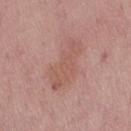- biopsy status — total-body-photography surveillance lesion; no biopsy
- location — the right thigh
- acquisition — total-body-photography crop, ~15 mm field of view
- subject — female, aged approximately 65
- tile lighting — white-light
- size — ~6.5 mm (longest diameter)